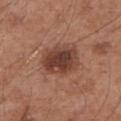This lesion was catalogued during total-body skin photography and was not selected for biopsy. Located on the left upper arm. The patient is a male aged approximately 55. A 15 mm crop from a total-body photograph taken for skin-cancer surveillance.